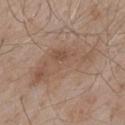notes — catalogued during a skin exam; not biopsied | imaging modality — total-body-photography crop, ~15 mm field of view | illumination — white-light | subject — male, aged approximately 55 | body site — the mid back.